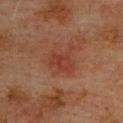Notes:
- notes · no biopsy performed (imaged during a skin exam)
- imaging modality · 15 mm crop, total-body photography
- lesion size · about 2.5 mm
- lighting · cross-polarized illumination
- image-analysis metrics · an automated nevus-likeness rating near 0 out of 100 and lesion-presence confidence of about 100/100
- patient · male, aged 73–77
- location · the upper back